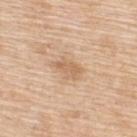Assessment:
Recorded during total-body skin imaging; not selected for excision or biopsy.
Acquisition and patient details:
The subject is a female approximately 55 years of age. A roughly 15 mm field-of-view crop from a total-body skin photograph. Automated tile analysis of the lesion measured a footprint of about 5.5 mm², a shape eccentricity near 0.85, and a symmetry-axis asymmetry near 0.3. It also reported a lesion color around L≈64 a*≈18 b*≈35 in CIELAB, a lesion–skin lightness drop of about 9, and a normalized lesion–skin contrast near 6. The lesion is on the back.